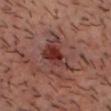Q: Was this lesion biopsied?
A: no biopsy performed (imaged during a skin exam)
Q: How was the tile lit?
A: cross-polarized illumination
Q: What kind of image is this?
A: ~15 mm crop, total-body skin-cancer survey
Q: Where on the body is the lesion?
A: the head or neck
Q: Who is the patient?
A: male, aged 38 to 42
Q: Lesion size?
A: ≈5.5 mm